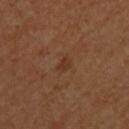Captured during whole-body skin photography for melanoma surveillance; the lesion was not biopsied. The patient is a male roughly 40 years of age. The tile uses cross-polarized illumination. The lesion is on the upper back. A 15 mm close-up tile from a total-body photography series done for melanoma screening.